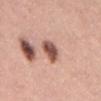Case summary:
• notes — imaged on a skin check; not biopsied